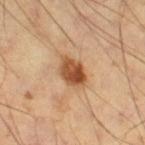illumination — cross-polarized; patient — male, aged 53 to 57; automated metrics — a classifier nevus-likeness of about 100/100 and lesion-presence confidence of about 100/100; image — 15 mm crop, total-body photography; body site — the right thigh.